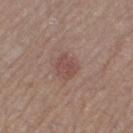Clinical impression: Imaged during a routine full-body skin examination; the lesion was not biopsied and no histopathology is available. Image and clinical context: A 15 mm close-up extracted from a 3D total-body photography capture. A female subject, aged around 50. The lesion is on the left thigh.